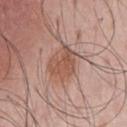The lesion was tiled from a total-body skin photograph and was not biopsied. A lesion tile, about 15 mm wide, cut from a 3D total-body photograph. The subject is a male in their 60s. The lesion is on the chest.